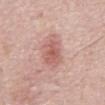Recorded during total-body skin imaging; not selected for excision or biopsy. The tile uses white-light illumination. A male patient, about 70 years old. Longest diameter approximately 5 mm. Located on the abdomen. A 15 mm close-up tile from a total-body photography series done for melanoma screening.